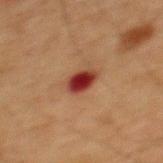| field | value |
|---|---|
| notes | catalogued during a skin exam; not biopsied |
| automated lesion analysis | a lesion color around L≈31 a*≈28 b*≈26 in CIELAB, a lesion–skin lightness drop of about 15, and a normalized border contrast of about 13; border irregularity of about 1.5 on a 0–10 scale, a color-variation rating of about 4.5/10, and a peripheral color-asymmetry measure near 1.5 |
| acquisition | 15 mm crop, total-body photography |
| subject | male, about 65 years old |
| diameter | ≈3 mm |
| illumination | cross-polarized |
| location | the mid back |